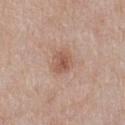The lesion was photographed on a routine skin check and not biopsied; there is no pathology result. Located on the right thigh. Measured at roughly 2.5 mm in maximum diameter. A female patient aged 58 to 62. This image is a 15 mm lesion crop taken from a total-body photograph.